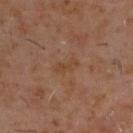The lesion was tiled from a total-body skin photograph and was not biopsied.